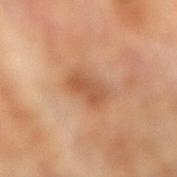Captured during whole-body skin photography for melanoma surveillance; the lesion was not biopsied. The recorded lesion diameter is about 3.5 mm. The lesion is on the right forearm. A region of skin cropped from a whole-body photographic capture, roughly 15 mm wide. Imaged with cross-polarized lighting. A female patient aged around 75.